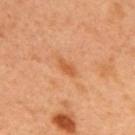The lesion was tiled from a total-body skin photograph and was not biopsied.